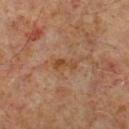Impression: The lesion was tiled from a total-body skin photograph and was not biopsied. Background: The lesion is on the right lower leg. Captured under cross-polarized illumination. The patient is a male about 60 years old. Automated image analysis of the tile measured an area of roughly 2.5 mm², a shape eccentricity near 0.95, and two-axis asymmetry of about 0.4. And it measured border irregularity of about 4.5 on a 0–10 scale, internal color variation of about 0 on a 0–10 scale, and peripheral color asymmetry of about 0. It also reported an automated nevus-likeness rating near 0 out of 100 and a detector confidence of about 100 out of 100 that the crop contains a lesion. A close-up tile cropped from a whole-body skin photograph, about 15 mm across. Measured at roughly 2.5 mm in maximum diameter.